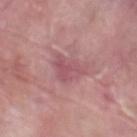The lesion is on the left lower leg. Cropped from a whole-body photographic skin survey; the tile spans about 15 mm. A male patient roughly 40 years of age.